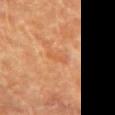  biopsy_status: not biopsied; imaged during a skin examination
  patient:
    sex: female
    age_approx: 80
  image:
    source: total-body photography crop
    field_of_view_mm: 15
  site: chest